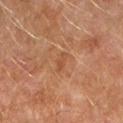biopsy_status: not biopsied; imaged during a skin examination